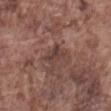The lesion was tiled from a total-body skin photograph and was not biopsied. The lesion is located on the abdomen. A lesion tile, about 15 mm wide, cut from a 3D total-body photograph. A male subject aged 73 to 77.